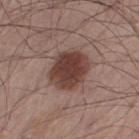Part of a total-body skin-imaging series; this lesion was reviewed on a skin check and was not flagged for biopsy. Cropped from a total-body skin-imaging series; the visible field is about 15 mm. The lesion is located on the leg. A male subject, in their mid- to late 50s. Imaged with white-light lighting. Approximately 4.5 mm at its widest.